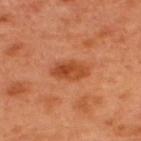Impression:
No biopsy was performed on this lesion — it was imaged during a full skin examination and was not determined to be concerning.
Clinical summary:
A roughly 15 mm field-of-view crop from a total-body skin photograph. A male patient, roughly 50 years of age. From the upper back.